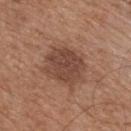Measured at roughly 4.5 mm in maximum diameter. A male patient, about 65 years old. The lesion is located on the mid back. A close-up tile cropped from a whole-body skin photograph, about 15 mm across. Automated image analysis of the tile measured a lesion area of about 14 mm² and an eccentricity of roughly 0.5. And it measured a border-irregularity index near 2/10, a within-lesion color-variation index near 2.5/10, and peripheral color asymmetry of about 1. And it measured an automated nevus-likeness rating near 25 out of 100 and lesion-presence confidence of about 100/100. Imaged with white-light lighting.